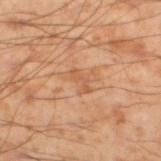The lesion was photographed on a routine skin check and not biopsied; there is no pathology result. Cropped from a total-body skin-imaging series; the visible field is about 15 mm. The patient is a male aged 53–57. Longest diameter approximately 2.5 mm. Imaged with cross-polarized lighting. Located on the left lower leg.